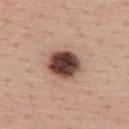Q: Is there a histopathology result?
A: total-body-photography surveillance lesion; no biopsy
Q: Lesion size?
A: ~4 mm (longest diameter)
Q: What is the anatomic site?
A: the upper back
Q: What are the patient's age and sex?
A: male, roughly 55 years of age
Q: Automated lesion metrics?
A: a footprint of about 11 mm² and two-axis asymmetry of about 0.1; an average lesion color of about L≈43 a*≈20 b*≈24 (CIELAB), about 23 CIELAB-L* units darker than the surrounding skin, and a normalized border contrast of about 15.5; a border-irregularity rating of about 1/10
Q: What is the imaging modality?
A: 15 mm crop, total-body photography